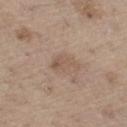{"biopsy_status": "not biopsied; imaged during a skin examination", "lighting": "white-light", "image": {"source": "total-body photography crop", "field_of_view_mm": 15}, "site": "left thigh", "patient": {"sex": "male", "age_approx": 70}}